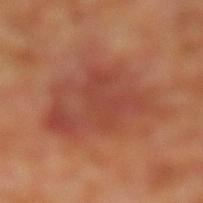Assessment: The lesion was tiled from a total-body skin photograph and was not biopsied. Image and clinical context: A close-up tile cropped from a whole-body skin photograph, about 15 mm across. On the left lower leg. A male subject, aged approximately 60.